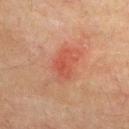This lesion was catalogued during total-body skin photography and was not selected for biopsy.
This image is a 15 mm lesion crop taken from a total-body photograph.
The lesion is on the upper back.
A male subject in their mid- to late 70s.